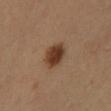The lesion was tiled from a total-body skin photograph and was not biopsied. A female subject, aged 53 to 57. Approximately 4 mm at its widest. Automated tile analysis of the lesion measured an eccentricity of roughly 0.8 and a shape-asymmetry score of about 0.1 (0 = symmetric). The software also gave an average lesion color of about L≈39 a*≈20 b*≈30 (CIELAB) and roughly 14 lightness units darker than nearby skin. And it measured a border-irregularity rating of about 1.5/10 and a within-lesion color-variation index near 3/10. On the left thigh. A lesion tile, about 15 mm wide, cut from a 3D total-body photograph.